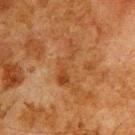Q: Is there a histopathology result?
A: total-body-photography surveillance lesion; no biopsy
Q: What is the imaging modality?
A: total-body-photography crop, ~15 mm field of view
Q: What is the anatomic site?
A: the upper back
Q: What are the patient's age and sex?
A: male, aged 78 to 82
Q: What did automated image analysis measure?
A: a footprint of about 7.5 mm² and a shape-asymmetry score of about 0.8 (0 = symmetric); an average lesion color of about L≈36 a*≈21 b*≈33 (CIELAB) and a normalized lesion–skin contrast near 5.5; border irregularity of about 10 on a 0–10 scale, a within-lesion color-variation index near 2/10, and peripheral color asymmetry of about 0.5
Q: How large is the lesion?
A: ≈6 mm
Q: What lighting was used for the tile?
A: cross-polarized illumination